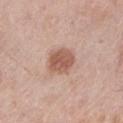{"biopsy_status": "not biopsied; imaged during a skin examination", "lighting": "white-light", "site": "left lower leg", "automated_metrics": {"area_mm2_approx": 9.5, "eccentricity": 0.55, "shape_asymmetry": 0.2, "cielab_L": 57, "cielab_a": 21, "cielab_b": 28, "vs_skin_darker_L": 12.0, "vs_skin_contrast_norm": 8.0, "nevus_likeness_0_100": 85, "lesion_detection_confidence_0_100": 100}, "patient": {"sex": "female", "age_approx": 65}, "image": {"source": "total-body photography crop", "field_of_view_mm": 15}}